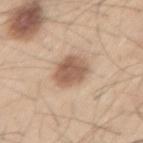<tbp_lesion>
<biopsy_status>not biopsied; imaged during a skin examination</biopsy_status>
<lesion_size>
  <long_diameter_mm_approx>4.0</long_diameter_mm_approx>
</lesion_size>
<patient>
  <sex>male</sex>
  <age_approx>60</age_approx>
</patient>
<automated_metrics>
  <eccentricity>0.7</eccentricity>
  <lesion_detection_confidence_0_100>100</lesion_detection_confidence_0_100>
</automated_metrics>
<lighting>white-light</lighting>
<image>
  <source>total-body photography crop</source>
  <field_of_view_mm>15</field_of_view_mm>
</image>
<site>left thigh</site>
</tbp_lesion>